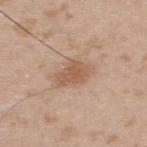Acquisition and patient details: This is a white-light tile. A 15 mm crop from a total-body photograph taken for skin-cancer surveillance. The patient is a male approximately 35 years of age. The lesion is located on the upper back. About 4 mm across. The lesion-visualizer software estimated a lesion area of about 7.5 mm², an outline eccentricity of about 0.8 (0 = round, 1 = elongated), and a symmetry-axis asymmetry near 0.25. The software also gave a lesion color around L≈57 a*≈19 b*≈31 in CIELAB, about 9 CIELAB-L* units darker than the surrounding skin, and a lesion-to-skin contrast of about 7 (normalized; higher = more distinct). The software also gave a nevus-likeness score of about 30/100 and lesion-presence confidence of about 100/100.